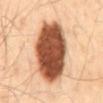{
  "biopsy_status": "not biopsied; imaged during a skin examination",
  "site": "mid back",
  "patient": {
    "sex": "male",
    "age_approx": 40
  },
  "image": {
    "source": "total-body photography crop",
    "field_of_view_mm": 15
  },
  "lesion_size": {
    "long_diameter_mm_approx": 8.5
  },
  "automated_metrics": {
    "area_mm2_approx": 36.0,
    "eccentricity": 0.8,
    "nevus_likeness_0_100": 100,
    "lesion_detection_confidence_0_100": 100
  }
}